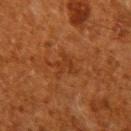{"biopsy_status": "not biopsied; imaged during a skin examination", "image": {"source": "total-body photography crop", "field_of_view_mm": 15}, "automated_metrics": {"eccentricity": 0.65, "shape_asymmetry": 0.55, "border_irregularity_0_10": 7.5, "color_variation_0_10": 0.0, "peripheral_color_asymmetry": 0.0, "nevus_likeness_0_100": 0}, "patient": {"sex": "male", "age_approx": 60}, "site": "right upper arm"}